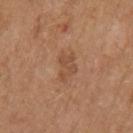This lesion was catalogued during total-body skin photography and was not selected for biopsy. A male subject, about 75 years old. Automated image analysis of the tile measured a lesion area of about 6 mm², an eccentricity of roughly 0.7, and two-axis asymmetry of about 0.35. The analysis additionally found a lesion color around L≈50 a*≈21 b*≈32 in CIELAB, about 7 CIELAB-L* units darker than the surrounding skin, and a lesion-to-skin contrast of about 5.5 (normalized; higher = more distinct). And it measured a within-lesion color-variation index near 2/10. The analysis additionally found a nevus-likeness score of about 5/100. Longest diameter approximately 3 mm. A roughly 15 mm field-of-view crop from a total-body skin photograph. Captured under white-light illumination. From the left upper arm.